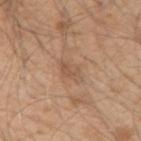Case summary:
• biopsy status · catalogued during a skin exam; not biopsied
• body site · the right upper arm
• lighting · white-light
• acquisition · ~15 mm tile from a whole-body skin photo
• lesion size · ≈3 mm
• subject · male, aged 48–52
• automated lesion analysis · an outline eccentricity of about 0.8 (0 = round, 1 = elongated) and a symmetry-axis asymmetry near 0.2; about 8 CIELAB-L* units darker than the surrounding skin and a lesion-to-skin contrast of about 5.5 (normalized; higher = more distinct); a nevus-likeness score of about 0/100 and a detector confidence of about 100 out of 100 that the crop contains a lesion